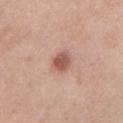The lesion was tiled from a total-body skin photograph and was not biopsied.
A female subject about 55 years old.
On the leg.
This is a white-light tile.
About 2.5 mm across.
The total-body-photography lesion software estimated a border-irregularity rating of about 2/10, a color-variation rating of about 3.5/10, and a peripheral color-asymmetry measure near 1.
A 15 mm crop from a total-body photograph taken for skin-cancer surveillance.